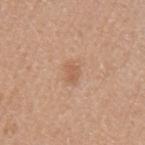Part of a total-body skin-imaging series; this lesion was reviewed on a skin check and was not flagged for biopsy. Automated tile analysis of the lesion measured a within-lesion color-variation index near 1/10 and a peripheral color-asymmetry measure near 0.5. It also reported an automated nevus-likeness rating near 15 out of 100. A close-up tile cropped from a whole-body skin photograph, about 15 mm across. Measured at roughly 2.5 mm in maximum diameter. Captured under white-light illumination. A male subject aged around 40. The lesion is on the arm.